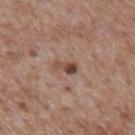Assessment:
The lesion was photographed on a routine skin check and not biopsied; there is no pathology result.
Background:
About 2.5 mm across. The lesion-visualizer software estimated an average lesion color of about L≈46 a*≈19 b*≈26 (CIELAB), about 12 CIELAB-L* units darker than the surrounding skin, and a lesion-to-skin contrast of about 9.5 (normalized; higher = more distinct). The analysis additionally found border irregularity of about 3.5 on a 0–10 scale, internal color variation of about 3.5 on a 0–10 scale, and a peripheral color-asymmetry measure near 0.5. A roughly 15 mm field-of-view crop from a total-body skin photograph. The subject is a male aged around 65. This is a white-light tile. Located on the mid back.